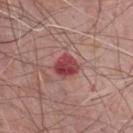Recorded during total-body skin imaging; not selected for excision or biopsy.
Cropped from a total-body skin-imaging series; the visible field is about 15 mm.
A male patient, aged 73 to 77.
On the front of the torso.